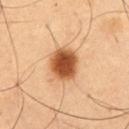Q: Is there a histopathology result?
A: total-body-photography surveillance lesion; no biopsy
Q: What did automated image analysis measure?
A: a footprint of about 11 mm², a shape eccentricity near 0.55, and a symmetry-axis asymmetry near 0.15; a border-irregularity index near 1/10, internal color variation of about 4.5 on a 0–10 scale, and radial color variation of about 1
Q: Where on the body is the lesion?
A: the chest
Q: How was this image acquired?
A: total-body-photography crop, ~15 mm field of view
Q: What lighting was used for the tile?
A: cross-polarized
Q: Patient demographics?
A: male, roughly 55 years of age
Q: What is the lesion's diameter?
A: about 4 mm